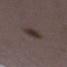notes — imaged on a skin check; not biopsied
acquisition — ~15 mm crop, total-body skin-cancer survey
location — the left lower leg
image-analysis metrics — an area of roughly 6.5 mm² and a shape-asymmetry score of about 0.15 (0 = symmetric)
size — about 3.5 mm
patient — female, aged approximately 30
tile lighting — white-light illumination